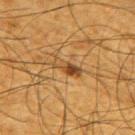{"biopsy_status": "not biopsied; imaged during a skin examination", "automated_metrics": {"area_mm2_approx": 4.5, "eccentricity": 0.95, "shape_asymmetry": 0.25, "border_irregularity_0_10": 3.5, "color_variation_0_10": 2.5, "peripheral_color_asymmetry": 0.5}, "lesion_size": {"long_diameter_mm_approx": 3.5}, "lighting": "cross-polarized", "site": "right upper arm", "image": {"source": "total-body photography crop", "field_of_view_mm": 15}, "patient": {"sex": "male", "age_approx": 65}}